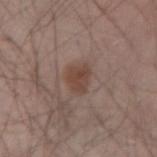Clinical impression: Recorded during total-body skin imaging; not selected for excision or biopsy. Background: From the left forearm. Approximately 3 mm at its widest. Cropped from a whole-body photographic skin survey; the tile spans about 15 mm. Automated image analysis of the tile measured a footprint of about 5.5 mm², an outline eccentricity of about 0.65 (0 = round, 1 = elongated), and two-axis asymmetry of about 0.3. And it measured an average lesion color of about L≈43 a*≈18 b*≈24 (CIELAB), roughly 8 lightness units darker than nearby skin, and a lesion-to-skin contrast of about 7.5 (normalized; higher = more distinct). The analysis additionally found an automated nevus-likeness rating near 85 out of 100 and a lesion-detection confidence of about 100/100. A male subject aged around 45.